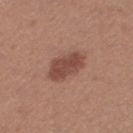Q: Is there a histopathology result?
A: no biopsy performed (imaged during a skin exam)
Q: How was this image acquired?
A: ~15 mm tile from a whole-body skin photo
Q: Who is the patient?
A: female, aged 38 to 42
Q: What is the anatomic site?
A: the right thigh